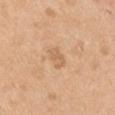workup: imaged on a skin check; not biopsied | subject: male, aged around 40 | image source: 15 mm crop, total-body photography | diameter: ≈3 mm | automated lesion analysis: a nevus-likeness score of about 0/100 | tile lighting: white-light illumination | body site: the left upper arm.